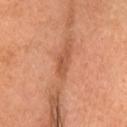workup = catalogued during a skin exam; not biopsied | image source = ~15 mm tile from a whole-body skin photo | patient = female, about 65 years old | body site = the head or neck.